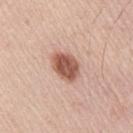Q: Was a biopsy performed?
A: no biopsy performed (imaged during a skin exam)
Q: What kind of image is this?
A: 15 mm crop, total-body photography
Q: What is the anatomic site?
A: the right upper arm
Q: Who is the patient?
A: male, aged 43 to 47
Q: What did automated image analysis measure?
A: a mean CIELAB color near L≈55 a*≈23 b*≈28, a lesion–skin lightness drop of about 17, and a lesion-to-skin contrast of about 10.5 (normalized; higher = more distinct); border irregularity of about 1.5 on a 0–10 scale, internal color variation of about 3.5 on a 0–10 scale, and peripheral color asymmetry of about 1